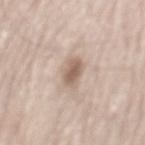workup = no biopsy performed (imaged during a skin exam)
tile lighting = white-light
subject = male, about 80 years old
diameter = ~3.5 mm (longest diameter)
location = the mid back
image = total-body-photography crop, ~15 mm field of view
TBP lesion metrics = an eccentricity of roughly 0.85; about 12 CIELAB-L* units darker than the surrounding skin